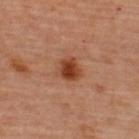workup: imaged on a skin check; not biopsied
anatomic site: the back
acquisition: 15 mm crop, total-body photography
patient: female, approximately 45 years of age